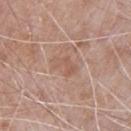Part of a total-body skin-imaging series; this lesion was reviewed on a skin check and was not flagged for biopsy. Captured under white-light illumination. From the chest. A 15 mm close-up tile from a total-body photography series done for melanoma screening. The recorded lesion diameter is about 3 mm. A male patient, roughly 65 years of age.